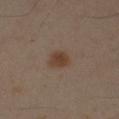workup: no biopsy performed (imaged during a skin exam)
anatomic site: the right upper arm
acquisition: ~15 mm crop, total-body skin-cancer survey
subject: male, aged 43–47
lighting: cross-polarized illumination
size: ≈3 mm
automated lesion analysis: a footprint of about 5 mm², an outline eccentricity of about 0.55 (0 = round, 1 = elongated), and a symmetry-axis asymmetry near 0.3; a mean CIELAB color near L≈38 a*≈15 b*≈26, about 8 CIELAB-L* units darker than the surrounding skin, and a normalized lesion–skin contrast near 8.5